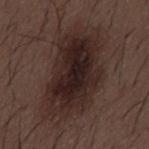Assessment:
The lesion was photographed on a routine skin check and not biopsied; there is no pathology result.
Background:
Imaged with white-light lighting. The lesion is located on the mid back. A lesion tile, about 15 mm wide, cut from a 3D total-body photograph. A male subject, approximately 50 years of age. Longest diameter approximately 9 mm.